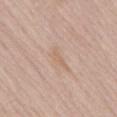Findings:
• workup — no biopsy performed (imaged during a skin exam)
• lesion size — about 3 mm
• illumination — white-light
• location — the lower back
• patient — male, approximately 70 years of age
• imaging modality — 15 mm crop, total-body photography